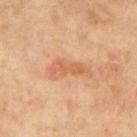Background:
From the back. The subject is a male roughly 70 years of age. Cropped from a whole-body photographic skin survey; the tile spans about 15 mm. Longest diameter approximately 4 mm.